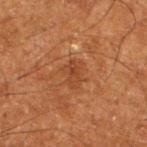workup: catalogued during a skin exam; not biopsied
location: the left lower leg
patient: male, roughly 65 years of age
lighting: cross-polarized
automated metrics: an area of roughly 5 mm²; an average lesion color of about L≈36 a*≈23 b*≈31 (CIELAB), a lesion–skin lightness drop of about 6, and a lesion-to-skin contrast of about 5 (normalized; higher = more distinct); a border-irregularity rating of about 3/10, a color-variation rating of about 3/10, and peripheral color asymmetry of about 1; an automated nevus-likeness rating near 0 out of 100 and lesion-presence confidence of about 100/100
image: ~15 mm crop, total-body skin-cancer survey